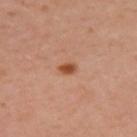biopsy status: no biopsy performed (imaged during a skin exam)
site: the arm
acquisition: total-body-photography crop, ~15 mm field of view
subject: female, approximately 40 years of age
lesion diameter: ~2 mm (longest diameter)
TBP lesion metrics: a lesion color around L≈50 a*≈26 b*≈35 in CIELAB, about 12 CIELAB-L* units darker than the surrounding skin, and a lesion-to-skin contrast of about 9.5 (normalized; higher = more distinct); a detector confidence of about 100 out of 100 that the crop contains a lesion